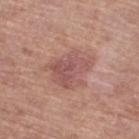workup: catalogued during a skin exam; not biopsied | imaging modality: ~15 mm crop, total-body skin-cancer survey | image-analysis metrics: a footprint of about 13 mm² and an outline eccentricity of about 0.55 (0 = round, 1 = elongated); a lesion color around L≈53 a*≈24 b*≈23 in CIELAB, about 8 CIELAB-L* units darker than the surrounding skin, and a normalized border contrast of about 6; a border-irregularity index near 5/10, a color-variation rating of about 3.5/10, and peripheral color asymmetry of about 1.5 | lighting: white-light | body site: the left lower leg | patient: female, aged approximately 75 | lesion diameter: about 5 mm.